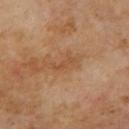Clinical impression: Recorded during total-body skin imaging; not selected for excision or biopsy. Context: The lesion is on the mid back. The recorded lesion diameter is about 3 mm. The tile uses cross-polarized illumination. Cropped from a total-body skin-imaging series; the visible field is about 15 mm. A male subject roughly 70 years of age. Automated tile analysis of the lesion measured a lesion area of about 2.5 mm² and a shape-asymmetry score of about 0.35 (0 = symmetric). The software also gave an average lesion color of about L≈48 a*≈22 b*≈35 (CIELAB) and a lesion–skin lightness drop of about 6. The software also gave a lesion-detection confidence of about 100/100.